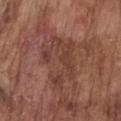The lesion was tiled from a total-body skin photograph and was not biopsied. The lesion is located on the chest. A 15 mm close-up tile from a total-body photography series done for melanoma screening. A male patient aged 73–77. The total-body-photography lesion software estimated a footprint of about 18 mm², a shape eccentricity near 0.75, and a shape-asymmetry score of about 0.55 (0 = symmetric). Imaged with white-light lighting.